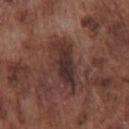biopsy status: total-body-photography surveillance lesion; no biopsy | site: the chest | size: about 6.5 mm | patient: male, approximately 75 years of age | image: total-body-photography crop, ~15 mm field of view.